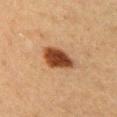follow-up: no biopsy performed (imaged during a skin exam)
acquisition: ~15 mm crop, total-body skin-cancer survey
lesion size: about 4.5 mm
TBP lesion metrics: an area of roughly 9.5 mm² and a symmetry-axis asymmetry near 0.2; an average lesion color of about L≈36 a*≈20 b*≈30 (CIELAB) and a lesion-to-skin contrast of about 12.5 (normalized; higher = more distinct); border irregularity of about 2 on a 0–10 scale, internal color variation of about 3.5 on a 0–10 scale, and peripheral color asymmetry of about 1; an automated nevus-likeness rating near 100 out of 100 and a detector confidence of about 100 out of 100 that the crop contains a lesion
subject: male, aged 48 to 52
anatomic site: the chest
lighting: cross-polarized illumination